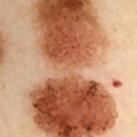Impression:
Captured during whole-body skin photography for melanoma surveillance; the lesion was not biopsied.
Clinical summary:
This is a cross-polarized tile. Cropped from a total-body skin-imaging series; the visible field is about 15 mm. The lesion is located on the left upper arm. The subject is a male aged approximately 55. The recorded lesion diameter is about 18.5 mm.